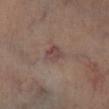No biopsy was performed on this lesion — it was imaged during a full skin examination and was not determined to be concerning. The lesion is on the right lower leg. A male patient roughly 70 years of age. Cropped from a whole-body photographic skin survey; the tile spans about 15 mm. Measured at roughly 2.5 mm in maximum diameter. Imaged with cross-polarized lighting. Automated image analysis of the tile measured a lesion area of about 4.5 mm², a shape eccentricity near 0.55, and two-axis asymmetry of about 0.25. The analysis additionally found a lesion color around L≈42 a*≈17 b*≈19 in CIELAB, about 7 CIELAB-L* units darker than the surrounding skin, and a lesion-to-skin contrast of about 6.5 (normalized; higher = more distinct).